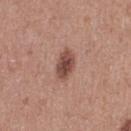<case>
  <biopsy_status>not biopsied; imaged during a skin examination</biopsy_status>
  <patient>
    <sex>female</sex>
    <age_approx>40</age_approx>
  </patient>
  <site>right thigh</site>
  <image>
    <source>total-body photography crop</source>
    <field_of_view_mm>15</field_of_view_mm>
  </image>
  <lighting>white-light</lighting>
</case>